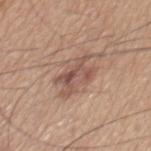biopsy status = total-body-photography surveillance lesion; no biopsy | diameter = ≈5.5 mm | automated lesion analysis = a footprint of about 12 mm², a shape eccentricity near 0.8, and a symmetry-axis asymmetry near 0.35; a border-irregularity rating of about 5.5/10, a within-lesion color-variation index near 6.5/10, and radial color variation of about 2; lesion-presence confidence of about 100/100 | patient = male, aged around 60 | anatomic site = the mid back | imaging modality = 15 mm crop, total-body photography.